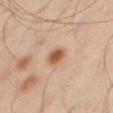biopsy_status: not biopsied; imaged during a skin examination
image:
  source: total-body photography crop
  field_of_view_mm: 15
patient:
  sex: male
  age_approx: 45
automated_metrics:
  area_mm2_approx: 4.5
  shape_asymmetry: 0.25
  nevus_likeness_0_100: 95
lighting: cross-polarized
site: right thigh